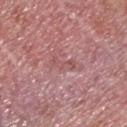Impression: The lesion was tiled from a total-body skin photograph and was not biopsied. Clinical summary: A 15 mm close-up tile from a total-body photography series done for melanoma screening. The lesion is located on the back. Longest diameter approximately 3 mm. A male patient roughly 75 years of age.